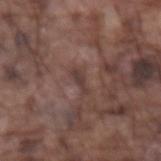Part of a total-body skin-imaging series; this lesion was reviewed on a skin check and was not flagged for biopsy.
A male subject in their mid- to late 70s.
From the left forearm.
Imaged with white-light lighting.
Longest diameter approximately 2.5 mm.
An algorithmic analysis of the crop reported a footprint of about 2.5 mm² and an eccentricity of roughly 0.9. And it measured a lesion color around L≈38 a*≈17 b*≈19 in CIELAB and about 7 CIELAB-L* units darker than the surrounding skin.
A roughly 15 mm field-of-view crop from a total-body skin photograph.